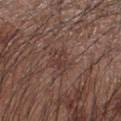This lesion was catalogued during total-body skin photography and was not selected for biopsy. The recorded lesion diameter is about 2.5 mm. A male patient approximately 65 years of age. A roughly 15 mm field-of-view crop from a total-body skin photograph. The lesion is located on the left forearm. The tile uses white-light illumination.